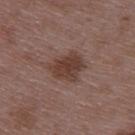biopsy status: total-body-photography surveillance lesion; no biopsy
image-analysis metrics: a shape-asymmetry score of about 0.25 (0 = symmetric); a nevus-likeness score of about 60/100 and a lesion-detection confidence of about 100/100
lesion size: ~4 mm (longest diameter)
patient: male, aged 48–52
acquisition: total-body-photography crop, ~15 mm field of view
anatomic site: the upper back
lighting: white-light illumination